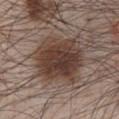biopsy_status: not biopsied; imaged during a skin examination
lighting: white-light
lesion_size:
  long_diameter_mm_approx: 6.5
site: chest
image:
  source: total-body photography crop
  field_of_view_mm: 15
patient:
  sex: male
  age_approx: 65